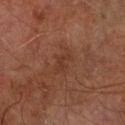Captured during whole-body skin photography for melanoma surveillance; the lesion was not biopsied. Approximately 2.5 mm at its widest. A male patient roughly 75 years of age. Cropped from a whole-body photographic skin survey; the tile spans about 15 mm. The lesion is located on the right forearm. This is a cross-polarized tile.